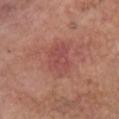| key | value |
|---|---|
| notes | imaged on a skin check; not biopsied |
| lesion size | about 4.5 mm |
| location | the chest |
| tile lighting | white-light |
| patient | female, in their mid- to late 60s |
| imaging modality | ~15 mm crop, total-body skin-cancer survey |